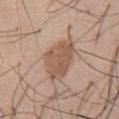Part of a total-body skin-imaging series; this lesion was reviewed on a skin check and was not flagged for biopsy.
From the abdomen.
Imaged with white-light lighting.
Measured at roughly 5.5 mm in maximum diameter.
A region of skin cropped from a whole-body photographic capture, roughly 15 mm wide.
A male patient roughly 55 years of age.
Automated tile analysis of the lesion measured an area of roughly 14 mm², an outline eccentricity of about 0.7 (0 = round, 1 = elongated), and two-axis asymmetry of about 0.2. It also reported a border-irregularity index near 2/10, internal color variation of about 2.5 on a 0–10 scale, and peripheral color asymmetry of about 1.